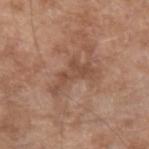imaging modality — ~15 mm tile from a whole-body skin photo
size — ~5.5 mm (longest diameter)
TBP lesion metrics — about 8 CIELAB-L* units darker than the surrounding skin and a normalized lesion–skin contrast near 6; a border-irregularity rating of about 8/10, a within-lesion color-variation index near 2/10, and peripheral color asymmetry of about 0.5; a classifier nevus-likeness of about 0/100 and a lesion-detection confidence of about 100/100
subject — male, aged around 60
lighting — white-light illumination
anatomic site — the arm